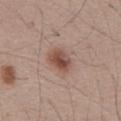The lesion-visualizer software estimated a footprint of about 6.5 mm², an eccentricity of roughly 0.65, and a shape-asymmetry score of about 0.2 (0 = symmetric). The software also gave an average lesion color of about L≈49 a*≈20 b*≈25 (CIELAB), a lesion–skin lightness drop of about 12, and a normalized border contrast of about 9. The software also gave a border-irregularity index near 1.5/10 and a within-lesion color-variation index near 5.5/10. The analysis additionally found an automated nevus-likeness rating near 95 out of 100 and a lesion-detection confidence of about 100/100. The lesion's longest dimension is about 3 mm. Imaged with white-light lighting. Located on the abdomen. A male subject aged 58–62. A 15 mm close-up tile from a total-body photography series done for melanoma screening.